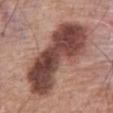The lesion was photographed on a routine skin check and not biopsied; there is no pathology result. The recorded lesion diameter is about 11.5 mm. From the abdomen. A 15 mm close-up extracted from a 3D total-body photography capture. The patient is a male aged 58 to 62.